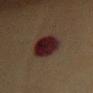biopsy status = catalogued during a skin exam; not biopsied | size = about 5.5 mm | location = the chest | image = total-body-photography crop, ~15 mm field of view | illumination = cross-polarized | automated metrics = a lesion area of about 13 mm²; a lesion color around L≈19 a*≈19 b*≈16 in CIELAB, a lesion–skin lightness drop of about 13, and a normalized border contrast of about 15.5; a border-irregularity index near 1.5/10, internal color variation of about 4 on a 0–10 scale, and a peripheral color-asymmetry measure near 1 | subject = female, in their mid- to late 50s.